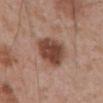No biopsy was performed on this lesion — it was imaged during a full skin examination and was not determined to be concerning.
From the abdomen.
A male patient aged approximately 70.
A region of skin cropped from a whole-body photographic capture, roughly 15 mm wide.